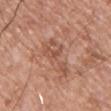Impression: Captured during whole-body skin photography for melanoma surveillance; the lesion was not biopsied. Context: Automated tile analysis of the lesion measured a lesion area of about 10 mm², an outline eccentricity of about 0.95 (0 = round, 1 = elongated), and a shape-asymmetry score of about 0.5 (0 = symmetric). The analysis additionally found an average lesion color of about L≈53 a*≈22 b*≈30 (CIELAB) and about 8 CIELAB-L* units darker than the surrounding skin. The software also gave a border-irregularity rating of about 7.5/10 and a within-lesion color-variation index near 4/10. This is a white-light tile. A 15 mm close-up extracted from a 3D total-body photography capture. A male subject roughly 65 years of age. The lesion's longest dimension is about 6.5 mm. The lesion is on the chest.